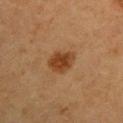Clinical impression:
The lesion was photographed on a routine skin check and not biopsied; there is no pathology result.
Acquisition and patient details:
Measured at roughly 3.5 mm in maximum diameter. Captured under cross-polarized illumination. An algorithmic analysis of the crop reported a lesion color around L≈35 a*≈20 b*≈32 in CIELAB, a lesion–skin lightness drop of about 10, and a lesion-to-skin contrast of about 9.5 (normalized; higher = more distinct). And it measured a border-irregularity index near 2.5/10, internal color variation of about 2.5 on a 0–10 scale, and peripheral color asymmetry of about 1. A 15 mm crop from a total-body photograph taken for skin-cancer surveillance. A female subject, aged 38 to 42. Located on the left upper arm.